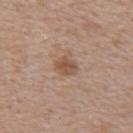Assessment:
The lesion was tiled from a total-body skin photograph and was not biopsied.
Background:
From the back. A female subject in their 50s. A 15 mm close-up extracted from a 3D total-body photography capture.